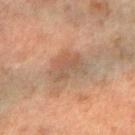Imaged during a routine full-body skin examination; the lesion was not biopsied and no histopathology is available. Located on the right forearm. A female subject in their mid-50s. Cropped from a whole-body photographic skin survey; the tile spans about 15 mm.